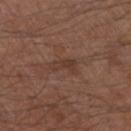| key | value |
|---|---|
| workup | catalogued during a skin exam; not biopsied |
| site | the left upper arm |
| lighting | white-light |
| acquisition | ~15 mm crop, total-body skin-cancer survey |
| subject | male, aged 48–52 |